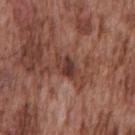Clinical impression:
Recorded during total-body skin imaging; not selected for excision or biopsy.
Background:
A male subject, aged approximately 75. On the chest. This is a white-light tile. A 15 mm close-up extracted from a 3D total-body photography capture. The lesion's longest dimension is about 3.5 mm.